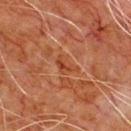The lesion was tiled from a total-body skin photograph and was not biopsied.
Cropped from a total-body skin-imaging series; the visible field is about 15 mm.
The lesion is on the chest.
The lesion's longest dimension is about 3 mm.
A male subject, about 80 years old.